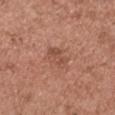<lesion>
  <biopsy_status>not biopsied; imaged during a skin examination</biopsy_status>
  <patient>
    <sex>male</sex>
    <age_approx>50</age_approx>
  </patient>
  <lighting>white-light</lighting>
  <automated_metrics>
    <border_irregularity_0_10>3.0</border_irregularity_0_10>
  </automated_metrics>
  <lesion_size>
    <long_diameter_mm_approx>2.5</long_diameter_mm_approx>
  </lesion_size>
  <site>head or neck</site>
  <image>
    <source>total-body photography crop</source>
    <field_of_view_mm>15</field_of_view_mm>
  </image>
</lesion>